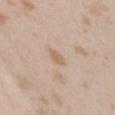biopsy_status: not biopsied; imaged during a skin examination
image:
  source: total-body photography crop
  field_of_view_mm: 15
automated_metrics:
  nevus_likeness_0_100: 0
  lesion_detection_confidence_0_100: 100
patient:
  sex: female
  age_approx: 25
site: front of the torso
lighting: white-light
lesion_size:
  long_diameter_mm_approx: 2.5